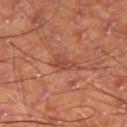{
  "biopsy_status": "not biopsied; imaged during a skin examination",
  "patient": {
    "sex": "male",
    "age_approx": 60
  },
  "image": {
    "source": "total-body photography crop",
    "field_of_view_mm": 15
  },
  "site": "right thigh"
}